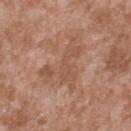follow-up — imaged on a skin check; not biopsied | image — 15 mm crop, total-body photography | location — the upper back | patient — male, approximately 45 years of age.